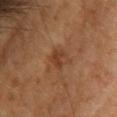Assessment: Captured during whole-body skin photography for melanoma surveillance; the lesion was not biopsied. Background: A female patient, roughly 70 years of age. A region of skin cropped from a whole-body photographic capture, roughly 15 mm wide. From the upper back.